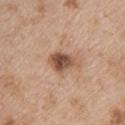The lesion was tiled from a total-body skin photograph and was not biopsied. Measured at roughly 4 mm in maximum diameter. A female patient aged approximately 45. The lesion is located on the upper back. This is a white-light tile. This image is a 15 mm lesion crop taken from a total-body photograph.